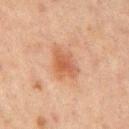<case>
<biopsy_status>not biopsied; imaged during a skin examination</biopsy_status>
<patient>
  <sex>male</sex>
  <age_approx>65</age_approx>
</patient>
<lesion_size>
  <long_diameter_mm_approx>4.0</long_diameter_mm_approx>
</lesion_size>
<image>
  <source>total-body photography crop</source>
  <field_of_view_mm>15</field_of_view_mm>
</image>
<automated_metrics>
  <area_mm2_approx>7.5</area_mm2_approx>
  <shape_asymmetry>0.3</shape_asymmetry>
  <cielab_L>47</cielab_L>
  <cielab_a>21</cielab_a>
  <cielab_b>30</cielab_b>
  <vs_skin_darker_L>8.0</vs_skin_darker_L>
  <vs_skin_contrast_norm>7.0</vs_skin_contrast_norm>
  <border_irregularity_0_10>3.5</border_irregularity_0_10>
  <peripheral_color_asymmetry>1.0</peripheral_color_asymmetry>
  <nevus_likeness_0_100>45</nevus_likeness_0_100>
</automated_metrics>
<lighting>cross-polarized</lighting>
<site>mid back</site>
</case>